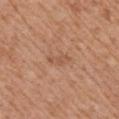biopsy status: total-body-photography surveillance lesion; no biopsy
image: ~15 mm tile from a whole-body skin photo
body site: the right upper arm
subject: male, roughly 75 years of age